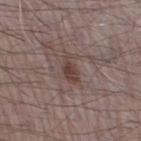Clinical impression:
Part of a total-body skin-imaging series; this lesion was reviewed on a skin check and was not flagged for biopsy.
Clinical summary:
The lesion is located on the right thigh. The lesion-visualizer software estimated a footprint of about 4.5 mm² and a symmetry-axis asymmetry near 0.3. It also reported a lesion color around L≈41 a*≈16 b*≈21 in CIELAB, about 9 CIELAB-L* units darker than the surrounding skin, and a normalized border contrast of about 8. And it measured a detector confidence of about 100 out of 100 that the crop contains a lesion. A 15 mm close-up tile from a total-body photography series done for melanoma screening. Measured at roughly 3.5 mm in maximum diameter. This is a white-light tile. The subject is a male aged approximately 70.